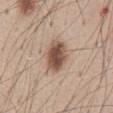Recorded during total-body skin imaging; not selected for excision or biopsy. From the front of the torso. A region of skin cropped from a whole-body photographic capture, roughly 15 mm wide. A male subject about 45 years old.